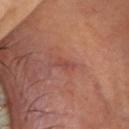follow-up=catalogued during a skin exam; not biopsied
image=~15 mm crop, total-body skin-cancer survey
subject=female, about 60 years old
body site=the head or neck